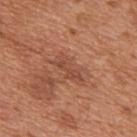Acquisition and patient details:
The lesion is located on the mid back. A roughly 15 mm field-of-view crop from a total-body skin photograph. The lesion-visualizer software estimated an area of roughly 5.5 mm², a shape eccentricity near 0.9, and a symmetry-axis asymmetry near 0.35. And it measured a lesion color around L≈48 a*≈25 b*≈31 in CIELAB and about 7 CIELAB-L* units darker than the surrounding skin. And it measured a border-irregularity index near 5/10, internal color variation of about 2 on a 0–10 scale, and a peripheral color-asymmetry measure near 0.5. The analysis additionally found a nevus-likeness score of about 0/100 and a detector confidence of about 100 out of 100 that the crop contains a lesion. A male patient, in their mid-60s. The lesion's longest dimension is about 4 mm.